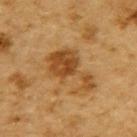biopsy status = total-body-photography surveillance lesion; no biopsy | patient = male, in their mid-80s or older | imaging modality = ~15 mm crop, total-body skin-cancer survey | lesion size = ≈6.5 mm | body site = the upper back.